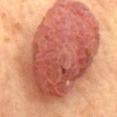Clinical impression: No biopsy was performed on this lesion — it was imaged during a full skin examination and was not determined to be concerning. Acquisition and patient details: On the mid back. The tile uses cross-polarized illumination. A female patient roughly 60 years of age. This image is a 15 mm lesion crop taken from a total-body photograph.